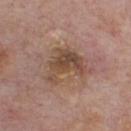biopsy status: no biopsy performed (imaged during a skin exam) | subject: male, in their mid-70s | diameter: ≈5.5 mm | location: the chest | imaging modality: ~15 mm tile from a whole-body skin photo.